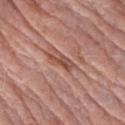– notes — no biopsy performed (imaged during a skin exam)
– body site — the arm
– automated metrics — a mean CIELAB color near L≈50 a*≈24 b*≈27, about 10 CIELAB-L* units darker than the surrounding skin, and a normalized lesion–skin contrast near 7; a within-lesion color-variation index near 3/10 and a peripheral color-asymmetry measure near 1
– patient — female, roughly 70 years of age
– image — ~15 mm tile from a whole-body skin photo
– tile lighting — white-light illumination